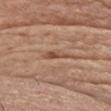biopsy status — imaged on a skin check; not biopsied | patient — male, approximately 65 years of age | lighting — white-light illumination | image — ~15 mm crop, total-body skin-cancer survey | automated metrics — an area of roughly 3.5 mm² and two-axis asymmetry of about 0.4 | anatomic site — the front of the torso.